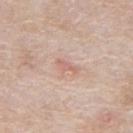{"biopsy_status": "not biopsied; imaged during a skin examination", "site": "front of the torso", "image": {"source": "total-body photography crop", "field_of_view_mm": 15}, "lighting": "white-light", "automated_metrics": {"nevus_likeness_0_100": 0, "lesion_detection_confidence_0_100": 100}, "patient": {"sex": "male", "age_approx": 65}, "lesion_size": {"long_diameter_mm_approx": 3.0}}